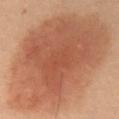Impression:
The lesion was photographed on a routine skin check and not biopsied; there is no pathology result.
Context:
Imaged with cross-polarized lighting. A 15 mm close-up tile from a total-body photography series done for melanoma screening. A male patient roughly 55 years of age. On the abdomen.